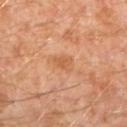follow-up = total-body-photography surveillance lesion; no biopsy
anatomic site = the left lower leg
acquisition = ~15 mm crop, total-body skin-cancer survey
TBP lesion metrics = a symmetry-axis asymmetry near 0.35; a border-irregularity index near 3/10 and peripheral color asymmetry of about 0.5
subject = male, aged approximately 30
tile lighting = cross-polarized
lesion diameter = ~3 mm (longest diameter)